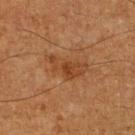Q: Was this lesion biopsied?
A: imaged on a skin check; not biopsied
Q: Patient demographics?
A: male, in their mid-60s
Q: How was this image acquired?
A: total-body-photography crop, ~15 mm field of view
Q: What is the anatomic site?
A: the right lower leg